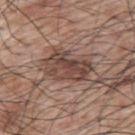notes=total-body-photography surveillance lesion; no biopsy | acquisition=15 mm crop, total-body photography | site=the back | patient=male, aged 68 to 72.